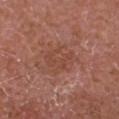The lesion was tiled from a total-body skin photograph and was not biopsied. A male patient about 75 years old. Located on the head or neck. A 15 mm close-up tile from a total-body photography series done for melanoma screening.